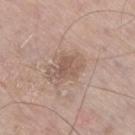– follow-up — imaged on a skin check; not biopsied
– body site — the right thigh
– subject — male, in their mid-70s
– image source — 15 mm crop, total-body photography